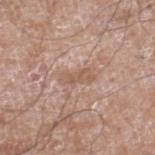| feature | finding |
|---|---|
| notes | no biopsy performed (imaged during a skin exam) |
| illumination | white-light |
| subject | male, aged around 60 |
| size | ≈3.5 mm |
| location | the right lower leg |
| acquisition | 15 mm crop, total-body photography |